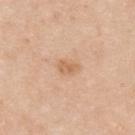<case>
<biopsy_status>not biopsied; imaged during a skin examination</biopsy_status>
<image>
  <source>total-body photography crop</source>
  <field_of_view_mm>15</field_of_view_mm>
</image>
<lesion_size>
  <long_diameter_mm_approx>2.5</long_diameter_mm_approx>
</lesion_size>
<patient>
  <sex>male</sex>
  <age_approx>35</age_approx>
</patient>
<lighting>white-light</lighting>
<automated_metrics>
  <vs_skin_darker_L>9.0</vs_skin_darker_L>
  <vs_skin_contrast_norm>6.0</vs_skin_contrast_norm>
  <border_irregularity_0_10>2.5</border_irregularity_0_10>
  <color_variation_0_10>2.0</color_variation_0_10>
  <peripheral_color_asymmetry>1.0</peripheral_color_asymmetry>
</automated_metrics>
<site>upper back</site>
</case>